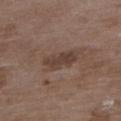workup: catalogued during a skin exam; not biopsied | tile lighting: white-light illumination | patient: female, in their 70s | image source: 15 mm crop, total-body photography | TBP lesion metrics: a lesion area of about 6.5 mm², an outline eccentricity of about 0.85 (0 = round, 1 = elongated), and two-axis asymmetry of about 0.2; a mean CIELAB color near L≈40 a*≈16 b*≈23, a lesion–skin lightness drop of about 8, and a normalized border contrast of about 7; a border-irregularity index near 3/10 and a within-lesion color-variation index near 2/10 | diameter: ≈4 mm | anatomic site: the upper back.